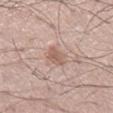Imaged during a routine full-body skin examination; the lesion was not biopsied and no histopathology is available. A male subject, aged approximately 55. Captured under white-light illumination. The lesion-visualizer software estimated a shape eccentricity near 0.85. And it measured a lesion color around L≈58 a*≈18 b*≈25 in CIELAB, about 9 CIELAB-L* units darker than the surrounding skin, and a normalized lesion–skin contrast near 6.5. The analysis additionally found border irregularity of about 2.5 on a 0–10 scale and radial color variation of about 0.5. The software also gave an automated nevus-likeness rating near 35 out of 100 and a lesion-detection confidence of about 100/100. A lesion tile, about 15 mm wide, cut from a 3D total-body photograph.